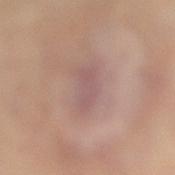workup: total-body-photography surveillance lesion; no biopsy
lighting: cross-polarized illumination
imaging modality: 15 mm crop, total-body photography
image-analysis metrics: an average lesion color of about L≈55 a*≈19 b*≈20 (CIELAB), roughly 6 lightness units darker than nearby skin, and a lesion-to-skin contrast of about 5 (normalized; higher = more distinct); an automated nevus-likeness rating near 0 out of 100 and a detector confidence of about 95 out of 100 that the crop contains a lesion
body site: the left leg
subject: female, approximately 40 years of age